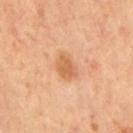The lesion was photographed on a routine skin check and not biopsied; there is no pathology result.
Cropped from a whole-body photographic skin survey; the tile spans about 15 mm.
This is a cross-polarized tile.
A male patient, aged approximately 70.
The lesion is located on the mid back.